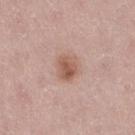Q: What kind of image is this?
A: ~15 mm crop, total-body skin-cancer survey
Q: Where on the body is the lesion?
A: the right thigh
Q: What are the patient's age and sex?
A: female, aged around 50